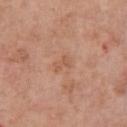This lesion was catalogued during total-body skin photography and was not selected for biopsy.
Automated image analysis of the tile measured an eccentricity of roughly 0.85. It also reported a lesion color around L≈57 a*≈22 b*≈32 in CIELAB, about 6 CIELAB-L* units darker than the surrounding skin, and a lesion-to-skin contrast of about 4.5 (normalized; higher = more distinct). And it measured border irregularity of about 3 on a 0–10 scale and internal color variation of about 1 on a 0–10 scale. The analysis additionally found an automated nevus-likeness rating near 0 out of 100 and lesion-presence confidence of about 100/100.
A male subject about 80 years old.
Located on the chest.
Measured at roughly 2.5 mm in maximum diameter.
Cropped from a total-body skin-imaging series; the visible field is about 15 mm.